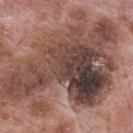Part of a total-body skin-imaging series; this lesion was reviewed on a skin check and was not flagged for biopsy. A region of skin cropped from a whole-body photographic capture, roughly 15 mm wide. Imaged with white-light lighting. Automated tile analysis of the lesion measured an area of roughly 80 mm² and a symmetry-axis asymmetry near 0.5. The software also gave about 14 CIELAB-L* units darker than the surrounding skin and a lesion-to-skin contrast of about 10 (normalized; higher = more distinct). And it measured border irregularity of about 7.5 on a 0–10 scale, a color-variation rating of about 10/10, and radial color variation of about 3.5. The analysis additionally found lesion-presence confidence of about 95/100. The lesion is located on the mid back. A male patient in their 70s.